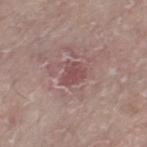{
  "biopsy_status": "not biopsied; imaged during a skin examination",
  "lesion_size": {
    "long_diameter_mm_approx": 2.5
  },
  "patient": {
    "sex": "female",
    "age_approx": "85+"
  },
  "lighting": "white-light",
  "automated_metrics": {
    "area_mm2_approx": 5.5,
    "eccentricity": 0.5,
    "border_irregularity_0_10": 2.5,
    "color_variation_0_10": 2.0,
    "peripheral_color_asymmetry": 0.5
  },
  "site": "leg",
  "image": {
    "source": "total-body photography crop",
    "field_of_view_mm": 15
  }
}